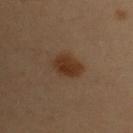Captured under cross-polarized illumination. About 3.5 mm across. On the chest. A region of skin cropped from a whole-body photographic capture, roughly 15 mm wide. The total-body-photography lesion software estimated a lesion color around L≈28 a*≈15 b*≈25 in CIELAB and a normalized lesion–skin contrast near 9. It also reported border irregularity of about 2 on a 0–10 scale, internal color variation of about 2.5 on a 0–10 scale, and peripheral color asymmetry of about 0.5. And it measured a nevus-likeness score of about 95/100 and a detector confidence of about 100 out of 100 that the crop contains a lesion. The subject is a female aged 48–52.